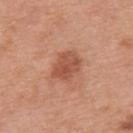Q: Patient demographics?
A: male, approximately 70 years of age
Q: Where on the body is the lesion?
A: the mid back
Q: How large is the lesion?
A: ≈4 mm
Q: How was this image acquired?
A: 15 mm crop, total-body photography
Q: How was the tile lit?
A: white-light illumination